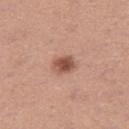Clinical impression: The lesion was photographed on a routine skin check and not biopsied; there is no pathology result. Acquisition and patient details: The lesion is on the right thigh. Automated image analysis of the tile measured an average lesion color of about L≈52 a*≈23 b*≈29 (CIELAB), a lesion–skin lightness drop of about 14, and a normalized lesion–skin contrast near 9. The analysis additionally found a border-irregularity index near 1.5/10. A female patient, aged 28 to 32. This image is a 15 mm lesion crop taken from a total-body photograph. Measured at roughly 3 mm in maximum diameter. This is a white-light tile.